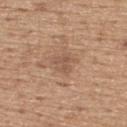Clinical impression:
Part of a total-body skin-imaging series; this lesion was reviewed on a skin check and was not flagged for biopsy.
Image and clinical context:
A close-up tile cropped from a whole-body skin photograph, about 15 mm across. The lesion-visualizer software estimated a lesion area of about 5 mm². It also reported border irregularity of about 5 on a 0–10 scale and a peripheral color-asymmetry measure near 1. A male subject, aged around 65. The lesion's longest dimension is about 3 mm. The lesion is located on the back.